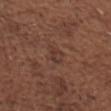Q: Was this lesion biopsied?
A: catalogued during a skin exam; not biopsied
Q: Lesion location?
A: the chest
Q: How was the tile lit?
A: white-light
Q: What is the imaging modality?
A: ~15 mm tile from a whole-body skin photo
Q: Patient demographics?
A: male, aged 73 to 77
Q: How large is the lesion?
A: ≈3 mm